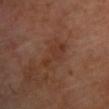Captured during whole-body skin photography for melanoma surveillance; the lesion was not biopsied.
On the front of the torso.
A male subject roughly 65 years of age.
This is a cross-polarized tile.
The lesion's longest dimension is about 5 mm.
This image is a 15 mm lesion crop taken from a total-body photograph.
The total-body-photography lesion software estimated a lesion area of about 6 mm², a shape eccentricity near 0.95, and two-axis asymmetry of about 0.45. It also reported a border-irregularity rating of about 6.5/10 and a within-lesion color-variation index near 2/10. The analysis additionally found an automated nevus-likeness rating near 0 out of 100.